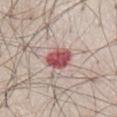Q: Who is the patient?
A: male, aged 78–82
Q: Where on the body is the lesion?
A: the chest
Q: What did automated image analysis measure?
A: a footprint of about 7.5 mm², an eccentricity of roughly 0.7, and two-axis asymmetry of about 0.15; a border-irregularity rating of about 1.5/10 and peripheral color asymmetry of about 1.5; a nevus-likeness score of about 0/100 and a lesion-detection confidence of about 100/100
Q: What kind of image is this?
A: ~15 mm tile from a whole-body skin photo